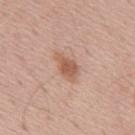<lesion>
  <biopsy_status>not biopsied; imaged during a skin examination</biopsy_status>
  <image>
    <source>total-body photography crop</source>
    <field_of_view_mm>15</field_of_view_mm>
  </image>
  <site>mid back</site>
  <patient>
    <sex>male</sex>
    <age_approx>55</age_approx>
  </patient>
</lesion>